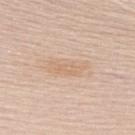Captured during whole-body skin photography for melanoma surveillance; the lesion was not biopsied. A male subject in their mid-60s. From the upper back. Captured under white-light illumination. Longest diameter approximately 4.5 mm. The total-body-photography lesion software estimated a nevus-likeness score of about 0/100. Cropped from a whole-body photographic skin survey; the tile spans about 15 mm.